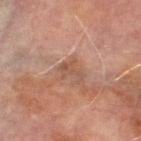| feature | finding |
|---|---|
| follow-up | catalogued during a skin exam; not biopsied |
| image source | total-body-photography crop, ~15 mm field of view |
| size | ~3 mm (longest diameter) |
| site | the right lower leg |
| patient | male, approximately 70 years of age |
| tile lighting | cross-polarized illumination |